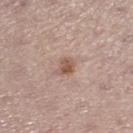tile lighting — white-light; subject — female, in their mid-60s; image — ~15 mm crop, total-body skin-cancer survey; lesion diameter — ~2.5 mm (longest diameter); location — the right lower leg.